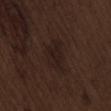This lesion was catalogued during total-body skin photography and was not selected for biopsy. The lesion is on the lower back. About 5 mm across. Automated tile analysis of the lesion measured about 4 CIELAB-L* units darker than the surrounding skin and a lesion-to-skin contrast of about 6.5 (normalized; higher = more distinct). The analysis additionally found a border-irregularity rating of about 4/10 and a peripheral color-asymmetry measure near 1. A roughly 15 mm field-of-view crop from a total-body skin photograph. A male patient about 70 years old.